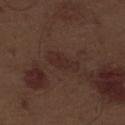Findings:
• follow-up: imaged on a skin check; not biopsied
• image: ~15 mm tile from a whole-body skin photo
• anatomic site: the front of the torso
• patient: male, aged around 70